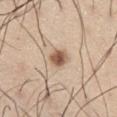workup: no biopsy performed (imaged during a skin exam) | image-analysis metrics: an area of roughly 4.5 mm², a shape eccentricity near 0.75, and a shape-asymmetry score of about 0.2 (0 = symmetric); a color-variation rating of about 3.5/10; a nevus-likeness score of about 95/100 and lesion-presence confidence of about 100/100 | site: the right thigh | patient: male, aged approximately 55 | diameter: about 3 mm | illumination: white-light | image source: ~15 mm tile from a whole-body skin photo.